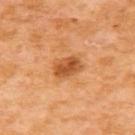This lesion was catalogued during total-body skin photography and was not selected for biopsy. Imaged with cross-polarized lighting. Automated image analysis of the tile measured an area of roughly 7.5 mm², an eccentricity of roughly 0.7, and a shape-asymmetry score of about 0.15 (0 = symmetric). And it measured an average lesion color of about L≈56 a*≈28 b*≈45 (CIELAB) and a lesion-to-skin contrast of about 8 (normalized; higher = more distinct). And it measured a border-irregularity rating of about 1.5/10 and a color-variation rating of about 5/10. A female patient approximately 55 years of age. A 15 mm close-up tile from a total-body photography series done for melanoma screening. Longest diameter approximately 3.5 mm. From the upper back.